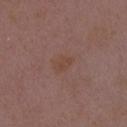Imaged during a routine full-body skin examination; the lesion was not biopsied and no histopathology is available. A 15 mm crop from a total-body photograph taken for skin-cancer surveillance. The subject is a female aged approximately 35. The lesion is on the chest.